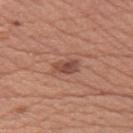<lesion>
  <biopsy_status>not biopsied; imaged during a skin examination</biopsy_status>
</lesion>